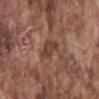Imaged during a routine full-body skin examination; the lesion was not biopsied and no histopathology is available. A male subject, aged around 75. The lesion-visualizer software estimated a footprint of about 5.5 mm². And it measured a lesion color around L≈41 a*≈19 b*≈25 in CIELAB, a lesion–skin lightness drop of about 8, and a lesion-to-skin contrast of about 6.5 (normalized; higher = more distinct). The analysis additionally found border irregularity of about 3 on a 0–10 scale and a color-variation rating of about 3/10. The software also gave a classifier nevus-likeness of about 0/100. Cropped from a whole-body photographic skin survey; the tile spans about 15 mm. From the mid back. Captured under white-light illumination.